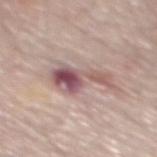Case summary:
* biopsy status — imaged on a skin check; not biopsied
* subject — male, aged 68–72
* tile lighting — white-light
* image — ~15 mm tile from a whole-body skin photo
* size — ~6.5 mm (longest diameter)
* image-analysis metrics — two-axis asymmetry of about 0.55; a nevus-likeness score of about 0/100
* body site — the back